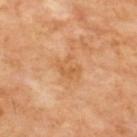| field | value |
|---|---|
| biopsy status | catalogued during a skin exam; not biopsied |
| imaging modality | total-body-photography crop, ~15 mm field of view |
| lesion size | about 2.5 mm |
| site | the upper back |
| automated metrics | an eccentricity of roughly 0.6 and a shape-asymmetry score of about 0.45 (0 = symmetric) |
| illumination | cross-polarized illumination |